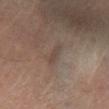  biopsy_status: not biopsied; imaged during a skin examination
  lighting: cross-polarized
  lesion_size:
    long_diameter_mm_approx: 2.5
  site: right lower leg
  patient:
    sex: male
    age_approx: 65
  image:
    source: total-body photography crop
    field_of_view_mm: 15